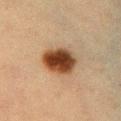Q: How large is the lesion?
A: ~4 mm (longest diameter)
Q: Who is the patient?
A: female, aged approximately 55
Q: Illumination type?
A: cross-polarized illumination
Q: Where on the body is the lesion?
A: the chest
Q: What kind of image is this?
A: ~15 mm tile from a whole-body skin photo